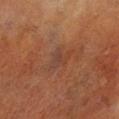{
  "biopsy_status": "not biopsied; imaged during a skin examination",
  "automated_metrics": {
    "cielab_L": 33,
    "cielab_a": 18,
    "cielab_b": 24,
    "vs_skin_darker_L": 4.0,
    "vs_skin_contrast_norm": 5.5,
    "border_irregularity_0_10": 5.5,
    "color_variation_0_10": 1.5
  },
  "image": {
    "source": "total-body photography crop",
    "field_of_view_mm": 15
  },
  "lesion_size": {
    "long_diameter_mm_approx": 3.5
  },
  "lighting": "cross-polarized",
  "patient": {
    "sex": "male",
    "age_approx": 70
  },
  "site": "right lower leg"
}